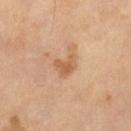| key | value |
|---|---|
| follow-up | imaged on a skin check; not biopsied |
| subject | male, aged 63–67 |
| image source | ~15 mm crop, total-body skin-cancer survey |
| diameter | ~2.5 mm (longest diameter) |
| tile lighting | cross-polarized |
| site | the left thigh |